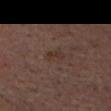biopsy_status: not biopsied; imaged during a skin examination
image:
  source: total-body photography crop
  field_of_view_mm: 15
lighting: white-light
site: head or neck
automated_metrics:
  eccentricity: 0.75
  border_irregularity_0_10: 2.0
  color_variation_0_10: 2.5
  nevus_likeness_0_100: 0
patient:
  sex: male
  age_approx: 75